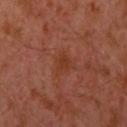tile lighting=cross-polarized illumination | acquisition=15 mm crop, total-body photography | location=the left upper arm | patient=male, aged 28 to 32.